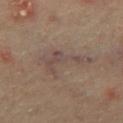| field | value |
|---|---|
| follow-up | total-body-photography surveillance lesion; no biopsy |
| lesion size | about 6 mm |
| illumination | cross-polarized illumination |
| patient | male, about 65 years old |
| site | the mid back |
| acquisition | 15 mm crop, total-body photography |